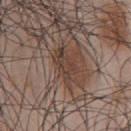The lesion was tiled from a total-body skin photograph and was not biopsied.
The subject is a male approximately 45 years of age.
A 15 mm close-up extracted from a 3D total-body photography capture.
Located on the chest.
Imaged with white-light lighting.